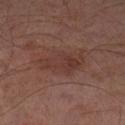<record>
<patient>
  <sex>male</sex>
  <age_approx>65</age_approx>
</patient>
<site>leg</site>
<image>
  <source>total-body photography crop</source>
  <field_of_view_mm>15</field_of_view_mm>
</image>
<lesion_size>
  <long_diameter_mm_approx>6.0</long_diameter_mm_approx>
</lesion_size>
</record>